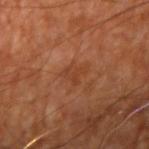notes: no biopsy performed (imaged during a skin exam) | lesion size: ≈3 mm | location: the right upper arm | TBP lesion metrics: an outline eccentricity of about 0.7 (0 = round, 1 = elongated); a border-irregularity index near 5/10, a within-lesion color-variation index near 1/10, and radial color variation of about 0; a classifier nevus-likeness of about 0/100 | patient: aged 63–67 | illumination: cross-polarized illumination | acquisition: total-body-photography crop, ~15 mm field of view.